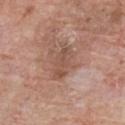follow-up: imaged on a skin check; not biopsied | image-analysis metrics: a lesion area of about 6.5 mm² and an outline eccentricity of about 0.9 (0 = round, 1 = elongated); a mean CIELAB color near L≈51 a*≈21 b*≈27 and a lesion-to-skin contrast of about 6 (normalized; higher = more distinct); a border-irregularity rating of about 3.5/10, internal color variation of about 3 on a 0–10 scale, and a peripheral color-asymmetry measure near 1 | tile lighting: white-light illumination | imaging modality: ~15 mm crop, total-body skin-cancer survey | anatomic site: the chest | patient: male, in their 60s.